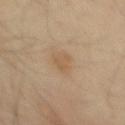Q: What did automated image analysis measure?
A: a lesion–skin lightness drop of about 6 and a lesion-to-skin contrast of about 5 (normalized; higher = more distinct)
Q: Patient demographics?
A: male, aged 53–57
Q: How large is the lesion?
A: ~3 mm (longest diameter)
Q: Lesion location?
A: the mid back
Q: How was this image acquired?
A: 15 mm crop, total-body photography
Q: How was the tile lit?
A: cross-polarized